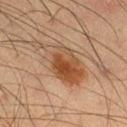<record>
<biopsy_status>not biopsied; imaged during a skin examination</biopsy_status>
<patient>
  <sex>male</sex>
  <age_approx>35</age_approx>
</patient>
<site>right thigh</site>
<lighting>cross-polarized</lighting>
<lesion_size>
  <long_diameter_mm_approx>7.0</long_diameter_mm_approx>
</lesion_size>
<image>
  <source>total-body photography crop</source>
  <field_of_view_mm>15</field_of_view_mm>
</image>
</record>